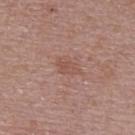Q: Was a biopsy performed?
A: total-body-photography surveillance lesion; no biopsy
Q: Patient demographics?
A: female, approximately 65 years of age
Q: What is the anatomic site?
A: the back
Q: What is the imaging modality?
A: ~15 mm tile from a whole-body skin photo
Q: Lesion size?
A: ≈2.5 mm